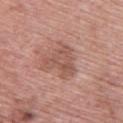Imaged during a routine full-body skin examination; the lesion was not biopsied and no histopathology is available.
The recorded lesion diameter is about 4 mm.
Located on the upper back.
Imaged with white-light lighting.
A male patient aged around 60.
This image is a 15 mm lesion crop taken from a total-body photograph.